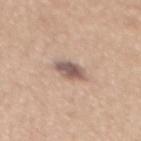* notes — imaged on a skin check; not biopsied
* image — total-body-photography crop, ~15 mm field of view
* location — the mid back
* patient — female, about 30 years old